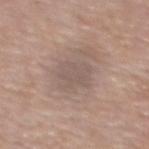Clinical impression: The lesion was photographed on a routine skin check and not biopsied; there is no pathology result. Image and clinical context: A roughly 15 mm field-of-view crop from a total-body skin photograph. On the upper back. A female subject approximately 55 years of age. The tile uses white-light illumination.